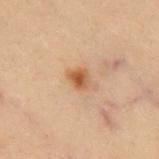Captured during whole-body skin photography for melanoma surveillance; the lesion was not biopsied. Automated image analysis of the tile measured a detector confidence of about 100 out of 100 that the crop contains a lesion. The patient is a male aged 53–57. Captured under cross-polarized illumination. On the chest. Longest diameter approximately 2.5 mm. A 15 mm close-up tile from a total-body photography series done for melanoma screening.